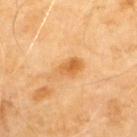Q: Is there a histopathology result?
A: total-body-photography surveillance lesion; no biopsy
Q: Lesion size?
A: ~4 mm (longest diameter)
Q: Where on the body is the lesion?
A: the upper back
Q: What are the patient's age and sex?
A: male, about 70 years old
Q: How was this image acquired?
A: total-body-photography crop, ~15 mm field of view
Q: Automated lesion metrics?
A: a shape eccentricity near 0.85 and a shape-asymmetry score of about 0.3 (0 = symmetric); roughly 10 lightness units darker than nearby skin and a normalized lesion–skin contrast near 7; an automated nevus-likeness rating near 50 out of 100
Q: Illumination type?
A: cross-polarized illumination